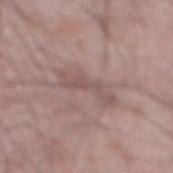<tbp_lesion>
<biopsy_status>not biopsied; imaged during a skin examination</biopsy_status>
<site>front of the torso</site>
<patient>
  <sex>male</sex>
  <age_approx>65</age_approx>
</patient>
<lighting>white-light</lighting>
<image>
  <source>total-body photography crop</source>
  <field_of_view_mm>15</field_of_view_mm>
</image>
<lesion_size>
  <long_diameter_mm_approx>5.0</long_diameter_mm_approx>
</lesion_size>
</tbp_lesion>